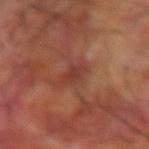follow-up: imaged on a skin check; not biopsied
location: the arm
subject: male, aged approximately 70
illumination: cross-polarized illumination
image source: ~15 mm tile from a whole-body skin photo